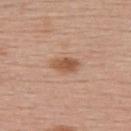The lesion was photographed on a routine skin check and not biopsied; there is no pathology result. A 15 mm close-up extracted from a 3D total-body photography capture. The total-body-photography lesion software estimated a lesion area of about 5.5 mm², an eccentricity of roughly 0.8, and a shape-asymmetry score of about 0.25 (0 = symmetric). The analysis additionally found border irregularity of about 2.5 on a 0–10 scale, a color-variation rating of about 3.5/10, and peripheral color asymmetry of about 1. The software also gave a classifier nevus-likeness of about 90/100 and a detector confidence of about 100 out of 100 that the crop contains a lesion. Imaged with white-light lighting. The subject is a female about 55 years old. Longest diameter approximately 3.5 mm. Located on the upper back.